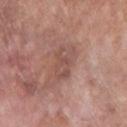Assessment:
Recorded during total-body skin imaging; not selected for excision or biopsy.
Image and clinical context:
Measured at roughly 4 mm in maximum diameter. The lesion is on the right upper arm. A 15 mm crop from a total-body photograph taken for skin-cancer surveillance. A male subject about 85 years old or older.